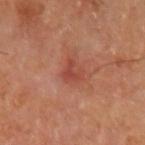Notes:
- image source: ~15 mm crop, total-body skin-cancer survey
- patient: male, aged 68 to 72
- illumination: cross-polarized illumination
- image-analysis metrics: roughly 8 lightness units darker than nearby skin and a normalized border contrast of about 6; a nevus-likeness score of about 5/100
- location: the left upper arm